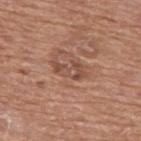Clinical impression: The lesion was photographed on a routine skin check and not biopsied; there is no pathology result. Image and clinical context: This image is a 15 mm lesion crop taken from a total-body photograph. A male patient roughly 75 years of age. The lesion is on the upper back. Approximately 4 mm at its widest. Captured under white-light illumination.